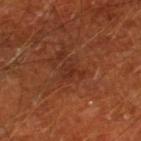{"biopsy_status": "not biopsied; imaged during a skin examination", "site": "right lower leg", "automated_metrics": {"area_mm2_approx": 3.5, "eccentricity": 0.7, "shape_asymmetry": 0.5, "cielab_L": 29, "cielab_a": 25, "cielab_b": 29, "vs_skin_darker_L": 5.0, "vs_skin_contrast_norm": 5.5, "nevus_likeness_0_100": 0, "lesion_detection_confidence_0_100": 100}, "image": {"source": "total-body photography crop", "field_of_view_mm": 15}, "lesion_size": {"long_diameter_mm_approx": 2.5}, "patient": {"sex": "male", "age_approx": 65}}